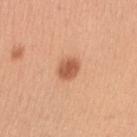workup: imaged on a skin check; not biopsied
subject: female, aged 28–32
automated lesion analysis: an area of roughly 5 mm², an eccentricity of roughly 0.5, and two-axis asymmetry of about 0.2; a lesion color around L≈58 a*≈26 b*≈35 in CIELAB and about 13 CIELAB-L* units darker than the surrounding skin; internal color variation of about 2 on a 0–10 scale and radial color variation of about 0.5; a lesion-detection confidence of about 100/100
anatomic site: the right upper arm
lighting: white-light
imaging modality: ~15 mm crop, total-body skin-cancer survey
diameter: ≈2.5 mm